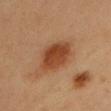workup = no biopsy performed (imaged during a skin exam)
image source = ~15 mm crop, total-body skin-cancer survey
subject = female, aged 33 to 37
diameter = about 5.5 mm
anatomic site = the head or neck